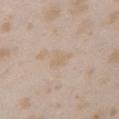The lesion's longest dimension is about 2.5 mm. A female patient about 25 years old. The tile uses white-light illumination. A lesion tile, about 15 mm wide, cut from a 3D total-body photograph. An algorithmic analysis of the crop reported roughly 4 lightness units darker than nearby skin and a lesion-to-skin contrast of about 4.5 (normalized; higher = more distinct). On the left upper arm.